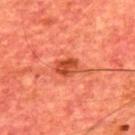Notes:
* workup · catalogued during a skin exam; not biopsied
* image · 15 mm crop, total-body photography
* tile lighting · cross-polarized illumination
* lesion size · ≈3 mm
* site · the upper back
* TBP lesion metrics · a lesion color around L≈46 a*≈36 b*≈39 in CIELAB, about 11 CIELAB-L* units darker than the surrounding skin, and a normalized border contrast of about 8; a lesion-detection confidence of about 100/100
* subject · male, aged approximately 65